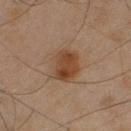{"automated_metrics": {"area_mm2_approx": 11.0, "eccentricity": 0.7, "cielab_L": 34, "cielab_a": 16, "cielab_b": 26, "vs_skin_darker_L": 8.0, "vs_skin_contrast_norm": 8.5, "border_irregularity_0_10": 1.5, "peripheral_color_asymmetry": 1.5, "lesion_detection_confidence_0_100": 100}, "image": {"source": "total-body photography crop", "field_of_view_mm": 15}, "site": "leg", "lighting": "cross-polarized", "lesion_size": {"long_diameter_mm_approx": 4.5}, "patient": {"sex": "male", "age_approx": 45}}